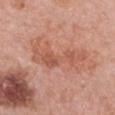Part of a total-body skin-imaging series; this lesion was reviewed on a skin check and was not flagged for biopsy. From the chest. Cropped from a total-body skin-imaging series; the visible field is about 15 mm. A female subject, roughly 60 years of age.